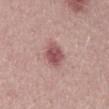No biopsy was performed on this lesion — it was imaged during a full skin examination and was not determined to be concerning.
A male patient, about 30 years old.
Approximately 3.5 mm at its widest.
A lesion tile, about 15 mm wide, cut from a 3D total-body photograph.
Automated tile analysis of the lesion measured a footprint of about 7 mm² and an outline eccentricity of about 0.7 (0 = round, 1 = elongated). It also reported an average lesion color of about L≈52 a*≈25 b*≈20 (CIELAB). The analysis additionally found a nevus-likeness score of about 65/100 and a lesion-detection confidence of about 100/100.
Captured under white-light illumination.